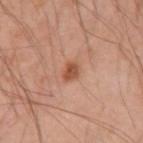Impression: The lesion was photographed on a routine skin check and not biopsied; there is no pathology result. Background: The lesion is on the left upper arm. Captured under cross-polarized illumination. A male subject in their mid-40s. Cropped from a whole-body photographic skin survey; the tile spans about 15 mm.